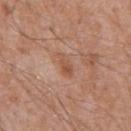notes = catalogued during a skin exam; not biopsied
acquisition = ~15 mm crop, total-body skin-cancer survey
size = ~3 mm (longest diameter)
patient = male, aged 58–62
lighting = white-light illumination
site = the upper back